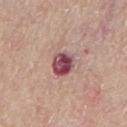Impression:
The lesion was photographed on a routine skin check and not biopsied; there is no pathology result.
Context:
A close-up tile cropped from a whole-body skin photograph, about 15 mm across. Automated tile analysis of the lesion measured a nevus-likeness score of about 0/100 and a detector confidence of about 100 out of 100 that the crop contains a lesion. The subject is a male aged 63 to 67. The lesion is located on the chest.